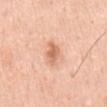The lesion was photographed on a routine skin check and not biopsied; there is no pathology result.